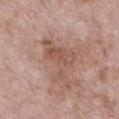workup: catalogued during a skin exam; not biopsied
acquisition: ~15 mm crop, total-body skin-cancer survey
illumination: white-light illumination
TBP lesion metrics: a mean CIELAB color near L≈54 a*≈19 b*≈27, a lesion–skin lightness drop of about 8, and a normalized border contrast of about 6; a border-irregularity index near 8/10, internal color variation of about 3.5 on a 0–10 scale, and peripheral color asymmetry of about 1.5; a lesion-detection confidence of about 100/100
patient: male, aged 48 to 52
location: the front of the torso